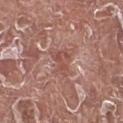notes: catalogued during a skin exam; not biopsied
location: the left lower leg
automated lesion analysis: an average lesion color of about L≈50 a*≈24 b*≈25 (CIELAB), about 8 CIELAB-L* units darker than the surrounding skin, and a lesion-to-skin contrast of about 6 (normalized; higher = more distinct)
image source: total-body-photography crop, ~15 mm field of view
diameter: ≈3 mm
subject: male, in their mid- to late 70s